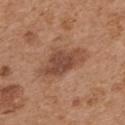Case summary:
* workup: catalogued during a skin exam; not biopsied
* patient: male, aged around 70
* location: the upper back
* tile lighting: white-light illumination
* acquisition: 15 mm crop, total-body photography
* diameter: ≈6 mm